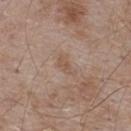Captured during whole-body skin photography for melanoma surveillance; the lesion was not biopsied. A male subject, approximately 55 years of age. The lesion is located on the upper back. A 15 mm crop from a total-body photograph taken for skin-cancer surveillance.